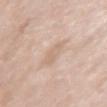subject = female, aged approximately 60 | image source = ~15 mm tile from a whole-body skin photo | anatomic site = the left upper arm.